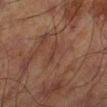patient:
  sex: male
  age_approx: 70
lighting: cross-polarized
site: left lower leg
automated_metrics:
  area_mm2_approx: 2.5
  eccentricity: 0.9
  shape_asymmetry: 0.35
  cielab_L: 31
  cielab_a: 18
  cielab_b: 21
  vs_skin_darker_L: 4.0
  vs_skin_contrast_norm: 4.5
  nevus_likeness_0_100: 0
  lesion_detection_confidence_0_100: 95
image:
  source: total-body photography crop
  field_of_view_mm: 15